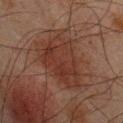No biopsy was performed on this lesion — it was imaged during a full skin examination and was not determined to be concerning. About 7.5 mm across. A male patient, aged approximately 65. Automated tile analysis of the lesion measured roughly 6 lightness units darker than nearby skin and a lesion-to-skin contrast of about 6.5 (normalized; higher = more distinct). The analysis additionally found peripheral color asymmetry of about 1. It also reported a nevus-likeness score of about 5/100 and a lesion-detection confidence of about 100/100. A region of skin cropped from a whole-body photographic capture, roughly 15 mm wide. The lesion is on the upper back.